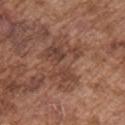Impression:
The lesion was photographed on a routine skin check and not biopsied; there is no pathology result.
Clinical summary:
The lesion is on the chest. The patient is a male in their mid- to late 70s. A 15 mm close-up tile from a total-body photography series done for melanoma screening. The tile uses white-light illumination. Measured at roughly 7 mm in maximum diameter.